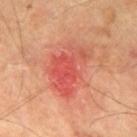The lesion was photographed on a routine skin check and not biopsied; there is no pathology result.
Captured under cross-polarized illumination.
A roughly 15 mm field-of-view crop from a total-body skin photograph.
Measured at roughly 6 mm in maximum diameter.
A male subject, roughly 70 years of age.
From the left thigh.